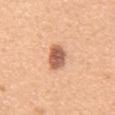notes = no biopsy performed (imaged during a skin exam)
image source = total-body-photography crop, ~15 mm field of view
subject = male, in their mid- to late 50s
site = the abdomen